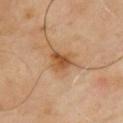Context:
A male patient, in their mid-60s. The lesion is located on the chest. Approximately 3 mm at its widest. Cropped from a whole-body photographic skin survey; the tile spans about 15 mm. The total-body-photography lesion software estimated a border-irregularity rating of about 3/10 and a peripheral color-asymmetry measure near 1.5. It also reported a classifier nevus-likeness of about 60/100. Imaged with cross-polarized lighting.